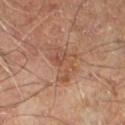Recorded during total-body skin imaging; not selected for excision or biopsy.
The lesion is on the left lower leg.
Longest diameter approximately 5 mm.
A male patient, in their 70s.
The tile uses cross-polarized illumination.
A close-up tile cropped from a whole-body skin photograph, about 15 mm across.